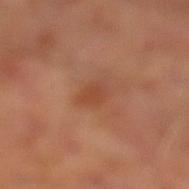follow-up: catalogued during a skin exam; not biopsied | image source: 15 mm crop, total-body photography | body site: the left lower leg | patient: male, aged approximately 65 | image-analysis metrics: a lesion–skin lightness drop of about 6 and a normalized border contrast of about 5; border irregularity of about 2.5 on a 0–10 scale and a peripheral color-asymmetry measure near 0.5.